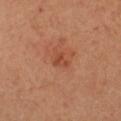Impression: Captured during whole-body skin photography for melanoma surveillance; the lesion was not biopsied. Image and clinical context: The tile uses cross-polarized illumination. This image is a 15 mm lesion crop taken from a total-body photograph. Longest diameter approximately 2 mm. A female patient aged around 40. The lesion is located on the chest. An algorithmic analysis of the crop reported peripheral color asymmetry of about 0. The software also gave a detector confidence of about 100 out of 100 that the crop contains a lesion.